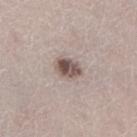Approximately 3 mm at its widest.
A female subject, approximately 50 years of age.
Automated image analysis of the tile measured a footprint of about 5.5 mm² and a shape-asymmetry score of about 0.25 (0 = symmetric). The software also gave a mean CIELAB color near L≈51 a*≈14 b*≈20, about 15 CIELAB-L* units darker than the surrounding skin, and a normalized border contrast of about 10.5.
A 15 mm crop from a total-body photograph taken for skin-cancer surveillance.
This is a white-light tile.
The lesion is located on the right lower leg.